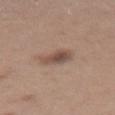The lesion was tiled from a total-body skin photograph and was not biopsied.